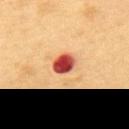follow-up=total-body-photography surveillance lesion; no biopsy | automated metrics=border irregularity of about 1.5 on a 0–10 scale, internal color variation of about 5.5 on a 0–10 scale, and peripheral color asymmetry of about 1.5; an automated nevus-likeness rating near 0 out of 100 and a lesion-detection confidence of about 100/100 | patient=male, approximately 55 years of age | location=the back | tile lighting=cross-polarized | imaging modality=total-body-photography crop, ~15 mm field of view | size=~3 mm (longest diameter).